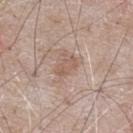The lesion was photographed on a routine skin check and not biopsied; there is no pathology result. A 15 mm crop from a total-body photograph taken for skin-cancer surveillance. The subject is a male aged 63 to 67. Imaged with white-light lighting. Measured at roughly 2.5 mm in maximum diameter. The total-body-photography lesion software estimated a border-irregularity rating of about 2/10. It also reported a nevus-likeness score of about 0/100. On the abdomen.